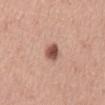biopsy status=no biopsy performed (imaged during a skin exam) | site=the abdomen | patient=male, aged around 50 | automated lesion analysis=a footprint of about 4 mm² and two-axis asymmetry of about 0.2; a color-variation rating of about 3/10 | acquisition=15 mm crop, total-body photography | illumination=white-light illumination.